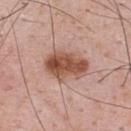No biopsy was performed on this lesion — it was imaged during a full skin examination and was not determined to be concerning.
The recorded lesion diameter is about 5 mm.
A male patient, aged 53 to 57.
On the upper back.
Cropped from a total-body skin-imaging series; the visible field is about 15 mm.
Captured under white-light illumination.